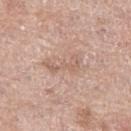| feature | finding |
|---|---|
| biopsy status | catalogued during a skin exam; not biopsied |
| diameter | ≈4.5 mm |
| automated lesion analysis | a mean CIELAB color near L≈61 a*≈18 b*≈27, roughly 8 lightness units darker than nearby skin, and a lesion-to-skin contrast of about 5 (normalized; higher = more distinct); border irregularity of about 6.5 on a 0–10 scale, a within-lesion color-variation index near 1/10, and a peripheral color-asymmetry measure near 0.5; a classifier nevus-likeness of about 0/100 and a lesion-detection confidence of about 100/100 |
| image source | ~15 mm tile from a whole-body skin photo |
| anatomic site | the left thigh |
| subject | female, in their mid-60s |
| illumination | white-light |